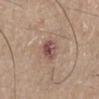Assessment: No biopsy was performed on this lesion — it was imaged during a full skin examination and was not determined to be concerning. Clinical summary: The recorded lesion diameter is about 2.5 mm. The lesion is on the right lower leg. A 15 mm crop from a total-body photograph taken for skin-cancer surveillance. A male subject, in their mid- to late 60s. An algorithmic analysis of the crop reported roughly 11 lightness units darker than nearby skin and a lesion-to-skin contrast of about 9 (normalized; higher = more distinct). It also reported an automated nevus-likeness rating near 15 out of 100 and a detector confidence of about 100 out of 100 that the crop contains a lesion.